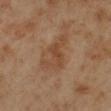The lesion was photographed on a routine skin check and not biopsied; there is no pathology result. A male subject, in their 70s. From the right lower leg. A lesion tile, about 15 mm wide, cut from a 3D total-body photograph. The tile uses cross-polarized illumination. The lesion's longest dimension is about 5 mm.